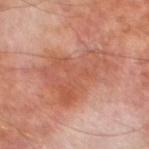Q: Is there a histopathology result?
A: no biopsy performed (imaged during a skin exam)
Q: What is the imaging modality?
A: 15 mm crop, total-body photography
Q: Who is the patient?
A: male, approximately 70 years of age
Q: Illumination type?
A: cross-polarized
Q: Where on the body is the lesion?
A: the left lower leg
Q: How large is the lesion?
A: ~8.5 mm (longest diameter)